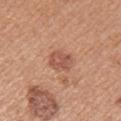A region of skin cropped from a whole-body photographic capture, roughly 15 mm wide. Automated image analysis of the tile measured a footprint of about 5.5 mm², a shape eccentricity near 0.6, and a symmetry-axis asymmetry near 0.25. The analysis additionally found an automated nevus-likeness rating near 40 out of 100 and lesion-presence confidence of about 100/100. A female subject aged 33 to 37. The tile uses white-light illumination. On the arm.